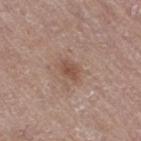Assessment:
Imaged during a routine full-body skin examination; the lesion was not biopsied and no histopathology is available.
Acquisition and patient details:
An algorithmic analysis of the crop reported a footprint of about 4 mm², an eccentricity of roughly 0.75, and a shape-asymmetry score of about 0.25 (0 = symmetric). It also reported a lesion color around L≈50 a*≈19 b*≈26 in CIELAB, a lesion–skin lightness drop of about 8, and a normalized lesion–skin contrast near 6.5. The software also gave a border-irregularity rating of about 2.5/10, a color-variation rating of about 2/10, and a peripheral color-asymmetry measure near 0.5. A female subject about 75 years old. Located on the right thigh. This image is a 15 mm lesion crop taken from a total-body photograph. About 2.5 mm across. Imaged with white-light lighting.